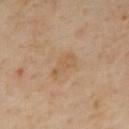body site: the mid back; patient: male, in their mid-60s; acquisition: total-body-photography crop, ~15 mm field of view.